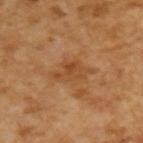workup: catalogued during a skin exam; not biopsied
image-analysis metrics: a mean CIELAB color near L≈45 a*≈22 b*≈37, a lesion–skin lightness drop of about 7, and a lesion-to-skin contrast of about 6 (normalized; higher = more distinct); a border-irregularity index near 4.5/10, internal color variation of about 4 on a 0–10 scale, and peripheral color asymmetry of about 1.5; a nevus-likeness score of about 0/100 and a detector confidence of about 100 out of 100 that the crop contains a lesion
acquisition: ~15 mm crop, total-body skin-cancer survey
lighting: cross-polarized
subject: male, aged 63 to 67
lesion diameter: ≈3.5 mm